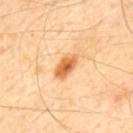| key | value |
|---|---|
| follow-up | imaged on a skin check; not biopsied |
| location | the mid back |
| lesion size | ~3.5 mm (longest diameter) |
| imaging modality | 15 mm crop, total-body photography |
| patient | male, aged 53 to 57 |